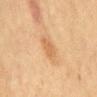follow-up: imaged on a skin check; not biopsied | TBP lesion metrics: a shape eccentricity near 0.9 and a shape-asymmetry score of about 0.3 (0 = symmetric); a mean CIELAB color near L≈56 a*≈19 b*≈37, about 7 CIELAB-L* units darker than the surrounding skin, and a normalized border contrast of about 6; border irregularity of about 3 on a 0–10 scale and a peripheral color-asymmetry measure near 0 | subject: female, aged 53–57 | image source: total-body-photography crop, ~15 mm field of view | anatomic site: the mid back | size: ≈3.5 mm | illumination: cross-polarized illumination.